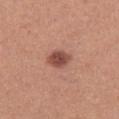| field | value |
|---|---|
| follow-up | no biopsy performed (imaged during a skin exam) |
| image | 15 mm crop, total-body photography |
| automated metrics | a footprint of about 5.5 mm², a shape eccentricity near 0.75, and a symmetry-axis asymmetry near 0.25; a border-irregularity index near 2/10 and a color-variation rating of about 3/10; a nevus-likeness score of about 95/100 and a detector confidence of about 100 out of 100 that the crop contains a lesion |
| subject | female, aged around 40 |
| body site | the leg |
| lighting | white-light |
| lesion size | ≈3 mm |